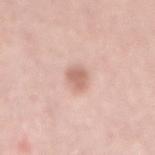Captured during whole-body skin photography for melanoma surveillance; the lesion was not biopsied.
Longest diameter approximately 3 mm.
An algorithmic analysis of the crop reported an area of roughly 4 mm², an outline eccentricity of about 0.75 (0 = round, 1 = elongated), and two-axis asymmetry of about 0.2. The software also gave a mean CIELAB color near L≈64 a*≈21 b*≈27, about 11 CIELAB-L* units darker than the surrounding skin, and a normalized lesion–skin contrast near 7.
A roughly 15 mm field-of-view crop from a total-body skin photograph.
From the mid back.
A female subject, approximately 55 years of age.
Captured under white-light illumination.